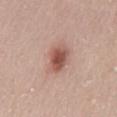Imaged during a routine full-body skin examination; the lesion was not biopsied and no histopathology is available. The lesion's longest dimension is about 4.5 mm. Automated image analysis of the tile measured an area of roughly 9.5 mm² and an eccentricity of roughly 0.8. It also reported border irregularity of about 2.5 on a 0–10 scale. It also reported a nevus-likeness score of about 90/100 and lesion-presence confidence of about 100/100. This image is a 15 mm lesion crop taken from a total-body photograph. From the mid back. A female patient aged approximately 30.